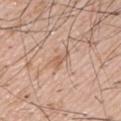subject: male, aged 53–57
size: ≈3 mm
image-analysis metrics: an area of roughly 3.5 mm² and an outline eccentricity of about 0.9 (0 = round, 1 = elongated); a mean CIELAB color near L≈60 a*≈19 b*≈31, about 8 CIELAB-L* units darker than the surrounding skin, and a lesion-to-skin contrast of about 5.5 (normalized; higher = more distinct); a border-irregularity index near 4/10 and peripheral color asymmetry of about 0; an automated nevus-likeness rating near 0 out of 100 and lesion-presence confidence of about 100/100
image: total-body-photography crop, ~15 mm field of view